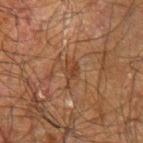This lesion was catalogued during total-body skin photography and was not selected for biopsy. A male patient in their 70s. This is a cross-polarized tile. A lesion tile, about 15 mm wide, cut from a 3D total-body photograph. The lesion is located on the right forearm. An algorithmic analysis of the crop reported a shape-asymmetry score of about 0.45 (0 = symmetric). The software also gave a classifier nevus-likeness of about 0/100 and lesion-presence confidence of about 65/100.